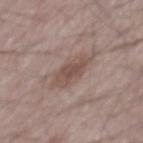This lesion was catalogued during total-body skin photography and was not selected for biopsy. Measured at roughly 4.5 mm in maximum diameter. A 15 mm crop from a total-body photograph taken for skin-cancer surveillance. Imaged with white-light lighting. The lesion is located on the mid back. A male patient, in their mid- to late 60s.